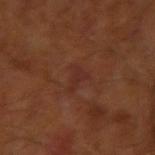biopsy_status: not biopsied; imaged during a skin examination
site: right upper arm
lighting: cross-polarized
automated_metrics:
  cielab_L: 27
  cielab_a: 22
  cielab_b: 24
  vs_skin_darker_L: 4.0
  vs_skin_contrast_norm: 5.0
  nevus_likeness_0_100: 0
  lesion_detection_confidence_0_100: 100
lesion_size:
  long_diameter_mm_approx: 2.5
patient:
  sex: male
  age_approx: 65
image:
  source: total-body photography crop
  field_of_view_mm: 15